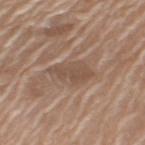Q: Is there a histopathology result?
A: imaged on a skin check; not biopsied
Q: Lesion location?
A: the left upper arm
Q: What lighting was used for the tile?
A: white-light illumination
Q: Lesion size?
A: ~5 mm (longest diameter)
Q: Who is the patient?
A: female, roughly 75 years of age
Q: How was this image acquired?
A: ~15 mm crop, total-body skin-cancer survey
Q: Automated lesion metrics?
A: a nevus-likeness score of about 0/100 and a lesion-detection confidence of about 55/100